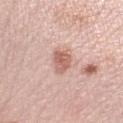Impression:
The lesion was tiled from a total-body skin photograph and was not biopsied.
Clinical summary:
From the left lower leg. Longest diameter approximately 3.5 mm. A roughly 15 mm field-of-view crop from a total-body skin photograph. A female subject, in their mid- to late 60s. Imaged with white-light lighting.